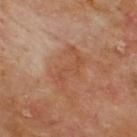Clinical impression:
Imaged during a routine full-body skin examination; the lesion was not biopsied and no histopathology is available.
Background:
A male subject roughly 70 years of age. From the upper back. A lesion tile, about 15 mm wide, cut from a 3D total-body photograph. Imaged with cross-polarized lighting.